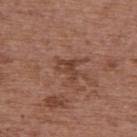Clinical summary: Imaged with white-light lighting. A female subject, aged approximately 65. The lesion-visualizer software estimated a lesion area of about 4.5 mm² and a shape eccentricity near 0.65. It also reported a border-irregularity rating of about 5.5/10 and a within-lesion color-variation index near 3.5/10. The software also gave a classifier nevus-likeness of about 0/100 and a detector confidence of about 100 out of 100 that the crop contains a lesion. Longest diameter approximately 3 mm. The lesion is on the upper back. This image is a 15 mm lesion crop taken from a total-body photograph.